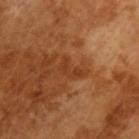A male patient aged 63–67. The total-body-photography lesion software estimated an average lesion color of about L≈38 a*≈26 b*≈36 (CIELAB), about 6 CIELAB-L* units darker than the surrounding skin, and a normalized lesion–skin contrast near 5.5. Longest diameter approximately 3 mm. Cropped from a whole-body photographic skin survey; the tile spans about 15 mm.